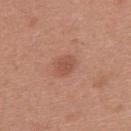<record>
<biopsy_status>not biopsied; imaged during a skin examination</biopsy_status>
<image>
  <source>total-body photography crop</source>
  <field_of_view_mm>15</field_of_view_mm>
</image>
<patient>
  <sex>female</sex>
  <age_approx>40</age_approx>
</patient>
<lighting>white-light</lighting>
<lesion_size>
  <long_diameter_mm_approx>2.5</long_diameter_mm_approx>
</lesion_size>
<site>upper back</site>
<automated_metrics>
  <cielab_L>52</cielab_L>
  <cielab_a>25</cielab_a>
  <cielab_b>29</cielab_b>
  <vs_skin_darker_L>8.0</vs_skin_darker_L>
  <border_irregularity_0_10>1.5</border_irregularity_0_10>
  <color_variation_0_10>2.0</color_variation_0_10>
  <peripheral_color_asymmetry>0.5</peripheral_color_asymmetry>
  <nevus_likeness_0_100>60</nevus_likeness_0_100>
</automated_metrics>
</record>